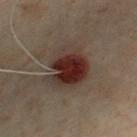Recorded during total-body skin imaging; not selected for excision or biopsy.
The tile uses cross-polarized illumination.
A 15 mm close-up extracted from a 3D total-body photography capture.
A male subject, about 50 years old.
The recorded lesion diameter is about 5.5 mm.
The lesion is on the chest.